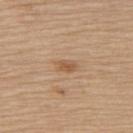Impression: Recorded during total-body skin imaging; not selected for excision or biopsy. Clinical summary: A female subject aged 63–67. The recorded lesion diameter is about 2.5 mm. This is a white-light tile. This image is a 15 mm lesion crop taken from a total-body photograph. The total-body-photography lesion software estimated a nevus-likeness score of about 25/100 and a lesion-detection confidence of about 100/100. Located on the upper back.